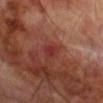follow-up: imaged on a skin check; not biopsied | patient: male, approximately 70 years of age | acquisition: ~15 mm tile from a whole-body skin photo | illumination: cross-polarized illumination | automated lesion analysis: a footprint of about 4 mm², a shape eccentricity near 0.7, and two-axis asymmetry of about 0.3; border irregularity of about 2.5 on a 0–10 scale, internal color variation of about 2 on a 0–10 scale, and radial color variation of about 0.5; a classifier nevus-likeness of about 0/100 and a lesion-detection confidence of about 100/100 | location: the right upper arm | diameter: ~2.5 mm (longest diameter).